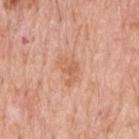Captured during whole-body skin photography for melanoma surveillance; the lesion was not biopsied. The tile uses white-light illumination. The total-body-photography lesion software estimated a nevus-likeness score of about 0/100 and lesion-presence confidence of about 100/100. A male patient approximately 70 years of age. Longest diameter approximately 3.5 mm. Located on the upper back. A 15 mm close-up tile from a total-body photography series done for melanoma screening.